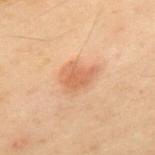Notes:
• notes: total-body-photography surveillance lesion; no biopsy
• acquisition: ~15 mm crop, total-body skin-cancer survey
• tile lighting: cross-polarized illumination
• site: the back
• patient: male, aged 53–57